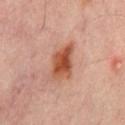Case summary:
– workup · no biopsy performed (imaged during a skin exam)
– subject · male, aged 63–67
– image source · ~15 mm tile from a whole-body skin photo
– lighting · cross-polarized illumination
– location · the lower back
– size · ~4.5 mm (longest diameter)